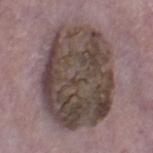This lesion was catalogued during total-body skin photography and was not selected for biopsy. Cropped from a total-body skin-imaging series; the visible field is about 15 mm. A male subject in their mid- to late 60s. About 11 mm across. The lesion is located on the leg. Captured under white-light illumination.